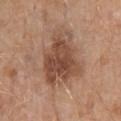notes: total-body-photography surveillance lesion; no biopsy | subject: male, in their 70s | site: the mid back | imaging modality: total-body-photography crop, ~15 mm field of view.